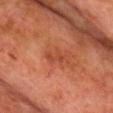{
  "biopsy_status": "not biopsied; imaged during a skin examination",
  "site": "chest",
  "automated_metrics": {
    "area_mm2_approx": 3.5,
    "eccentricity": 0.9,
    "shape_asymmetry": 0.35,
    "vs_skin_darker_L": 6.0,
    "vs_skin_contrast_norm": 5.0,
    "color_variation_0_10": 0.0,
    "peripheral_color_asymmetry": 0.0,
    "nevus_likeness_0_100": 0,
    "lesion_detection_confidence_0_100": 100
  },
  "lesion_size": {
    "long_diameter_mm_approx": 3.0
  },
  "patient": {
    "sex": "male",
    "age_approx": 75
  },
  "image": {
    "source": "total-body photography crop",
    "field_of_view_mm": 15
  },
  "lighting": "cross-polarized"
}